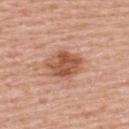biopsy_status: not biopsied; imaged during a skin examination
automated_metrics:
  vs_skin_contrast_norm: 8.5
  nevus_likeness_0_100: 85
site: upper back
patient:
  sex: female
  age_approx: 60
image:
  source: total-body photography crop
  field_of_view_mm: 15
lesion_size:
  long_diameter_mm_approx: 4.5